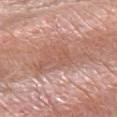{"biopsy_status": "not biopsied; imaged during a skin examination", "automated_metrics": {"nevus_likeness_0_100": 0, "lesion_detection_confidence_0_100": 90}, "patient": {"sex": "female", "age_approx": 40}, "image": {"source": "total-body photography crop", "field_of_view_mm": 15}, "site": "left forearm"}